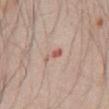Captured during whole-body skin photography for melanoma surveillance; the lesion was not biopsied.
A male subject, in their mid- to late 40s.
This image is a 15 mm lesion crop taken from a total-body photograph.
Located on the abdomen.
The recorded lesion diameter is about 3 mm.
The tile uses white-light illumination.
The lesion-visualizer software estimated a lesion color around L≈59 a*≈23 b*≈25 in CIELAB and about 10 CIELAB-L* units darker than the surrounding skin. It also reported an automated nevus-likeness rating near 0 out of 100.